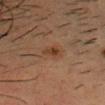Findings:
– follow-up: imaged on a skin check; not biopsied
– image-analysis metrics: a lesion color around L≈36 a*≈19 b*≈29 in CIELAB, about 7 CIELAB-L* units darker than the surrounding skin, and a normalized border contrast of about 7; radial color variation of about 1.5
– site: the head or neck
– illumination: cross-polarized
– lesion size: ~2.5 mm (longest diameter)
– subject: male, aged 33–37
– imaging modality: ~15 mm crop, total-body skin-cancer survey